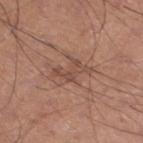{"biopsy_status": "not biopsied; imaged during a skin examination", "automated_metrics": {"area_mm2_approx": 5.5, "border_irregularity_0_10": 6.0, "peripheral_color_asymmetry": 1.0, "nevus_likeness_0_100": 0, "lesion_detection_confidence_0_100": 85}, "site": "leg", "patient": {"sex": "male", "age_approx": 55}, "image": {"source": "total-body photography crop", "field_of_view_mm": 15}}